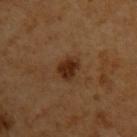Part of a total-body skin-imaging series; this lesion was reviewed on a skin check and was not flagged for biopsy.
Automated image analysis of the tile measured an eccentricity of roughly 0.7 and a shape-asymmetry score of about 0.25 (0 = symmetric). And it measured an average lesion color of about L≈29 a*≈20 b*≈31 (CIELAB), roughly 11 lightness units darker than nearby skin, and a lesion-to-skin contrast of about 10.5 (normalized; higher = more distinct).
A region of skin cropped from a whole-body photographic capture, roughly 15 mm wide.
The lesion is located on the left upper arm.
A female subject, aged approximately 55.
The tile uses cross-polarized illumination.
Measured at roughly 3 mm in maximum diameter.